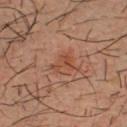Recorded during total-body skin imaging; not selected for excision or biopsy.
The lesion is located on the chest.
Imaged with cross-polarized lighting.
A male subject in their mid- to late 30s.
A 15 mm close-up tile from a total-body photography series done for melanoma screening.